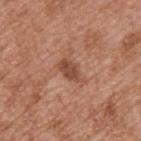follow-up: total-body-photography surveillance lesion; no biopsy | imaging modality: total-body-photography crop, ~15 mm field of view | lesion diameter: ≈3.5 mm | illumination: white-light | anatomic site: the upper back | patient: male, about 55 years old | automated lesion analysis: a footprint of about 5 mm² and an eccentricity of roughly 0.8; a mean CIELAB color near L≈48 a*≈24 b*≈31 and a lesion-to-skin contrast of about 7.5 (normalized; higher = more distinct).